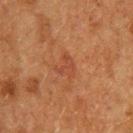Notes:
– follow-up — total-body-photography surveillance lesion; no biopsy
– automated lesion analysis — roughly 5 lightness units darker than nearby skin and a normalized lesion–skin contrast near 5; border irregularity of about 6 on a 0–10 scale, a color-variation rating of about 1.5/10, and peripheral color asymmetry of about 0.5
– site — the upper back
– tile lighting — cross-polarized illumination
– image source — 15 mm crop, total-body photography
– patient — male, aged 48 to 52